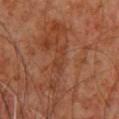Findings:
* notes: catalogued during a skin exam; not biopsied
* image-analysis metrics: a mean CIELAB color near L≈36 a*≈22 b*≈30 and roughly 5 lightness units darker than nearby skin; a detector confidence of about 90 out of 100 that the crop contains a lesion
* diameter: ~3.5 mm (longest diameter)
* acquisition: 15 mm crop, total-body photography
* illumination: cross-polarized illumination
* patient: male, aged around 60
* location: the back